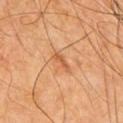This lesion was catalogued during total-body skin photography and was not selected for biopsy. A male patient in their mid-60s. A 15 mm close-up extracted from a 3D total-body photography capture. The lesion is located on the chest.